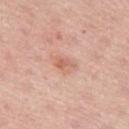This lesion was catalogued during total-body skin photography and was not selected for biopsy. The tile uses white-light illumination. The total-body-photography lesion software estimated about 8 CIELAB-L* units darker than the surrounding skin and a normalized border contrast of about 5.5. A roughly 15 mm field-of-view crop from a total-body skin photograph. The lesion is on the left thigh. The lesion's longest dimension is about 3 mm. The patient is a female in their mid-60s.